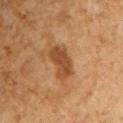Impression:
The lesion was tiled from a total-body skin photograph and was not biopsied.
Clinical summary:
The tile uses cross-polarized illumination. Automated image analysis of the tile measured a footprint of about 8.5 mm², an outline eccentricity of about 0.8 (0 = round, 1 = elongated), and a shape-asymmetry score of about 0.25 (0 = symmetric). The analysis additionally found an average lesion color of about L≈39 a*≈20 b*≈32 (CIELAB), a lesion–skin lightness drop of about 10, and a normalized border contrast of about 8. The software also gave an automated nevus-likeness rating near 25 out of 100 and lesion-presence confidence of about 100/100. The lesion is on the left upper arm. A male patient, aged 58 to 62. This image is a 15 mm lesion crop taken from a total-body photograph. The recorded lesion diameter is about 4 mm.